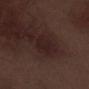lighting=white-light illumination | site=the right lower leg | image source=~15 mm tile from a whole-body skin photo | image-analysis metrics=an area of roughly 5.5 mm², a shape eccentricity near 0.5, and a symmetry-axis asymmetry near 0.15; a color-variation rating of about 1.5/10 and a peripheral color-asymmetry measure near 0.5; an automated nevus-likeness rating near 0 out of 100 and lesion-presence confidence of about 85/100 | patient=male, about 70 years old | size=about 3 mm.